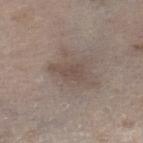Q: Was a biopsy performed?
A: total-body-photography surveillance lesion; no biopsy
Q: Automated lesion metrics?
A: an average lesion color of about L≈48 a*≈13 b*≈21 (CIELAB), a lesion–skin lightness drop of about 7, and a normalized border contrast of about 6; a nevus-likeness score of about 0/100 and lesion-presence confidence of about 100/100
Q: What kind of image is this?
A: total-body-photography crop, ~15 mm field of view
Q: What are the patient's age and sex?
A: male, aged 68–72
Q: How was the tile lit?
A: white-light
Q: Where on the body is the lesion?
A: the right lower leg